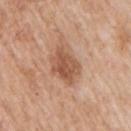workup=catalogued during a skin exam; not biopsied | site=the right upper arm | illumination=white-light | lesion size=about 4 mm | image source=~15 mm tile from a whole-body skin photo | patient=male, aged around 65 | automated metrics=a footprint of about 10 mm²; a nevus-likeness score of about 35/100 and lesion-presence confidence of about 100/100.